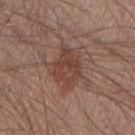This lesion was catalogued during total-body skin photography and was not selected for biopsy.
The lesion is on the right forearm.
The tile uses white-light illumination.
Longest diameter approximately 5 mm.
Cropped from a whole-body photographic skin survey; the tile spans about 15 mm.
A male patient, roughly 40 years of age.